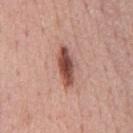{"biopsy_status": "not biopsied; imaged during a skin examination", "automated_metrics": {"cielab_L": 50, "cielab_a": 24, "cielab_b": 26, "vs_skin_darker_L": 16.0, "vs_skin_contrast_norm": 11.0, "border_irregularity_0_10": 3.0}, "lesion_size": {"long_diameter_mm_approx": 5.0}, "patient": {"sex": "male", "age_approx": 75}, "image": {"source": "total-body photography crop", "field_of_view_mm": 15}, "site": "chest"}